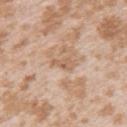Clinical impression: The lesion was tiled from a total-body skin photograph and was not biopsied. Acquisition and patient details: Measured at roughly 2.5 mm in maximum diameter. The lesion is located on the left upper arm. The subject is a female roughly 25 years of age. Imaged with white-light lighting. Automated image analysis of the tile measured a lesion area of about 3 mm², an outline eccentricity of about 0.85 (0 = round, 1 = elongated), and a shape-asymmetry score of about 0.35 (0 = symmetric). The analysis additionally found an average lesion color of about L≈61 a*≈18 b*≈32 (CIELAB), a lesion–skin lightness drop of about 8, and a lesion-to-skin contrast of about 6 (normalized; higher = more distinct). The software also gave border irregularity of about 3.5 on a 0–10 scale and a peripheral color-asymmetry measure near 0. The analysis additionally found a classifier nevus-likeness of about 0/100. A close-up tile cropped from a whole-body skin photograph, about 15 mm across.